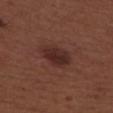The lesion was photographed on a routine skin check and not biopsied; there is no pathology result. Located on the back. The subject is a male aged 28–32. Cropped from a whole-body photographic skin survey; the tile spans about 15 mm. About 4 mm across.